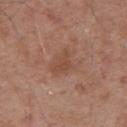biopsy_status: not biopsied; imaged during a skin examination
patient:
  sex: male
  age_approx: 55
lesion_size:
  long_diameter_mm_approx: 2.5
lighting: white-light
site: arm
image:
  source: total-body photography crop
  field_of_view_mm: 15